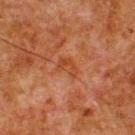Recorded during total-body skin imaging; not selected for excision or biopsy.
Located on the upper back.
The subject is a male about 80 years old.
A lesion tile, about 15 mm wide, cut from a 3D total-body photograph.